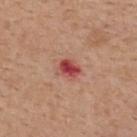Part of a total-body skin-imaging series; this lesion was reviewed on a skin check and was not flagged for biopsy.